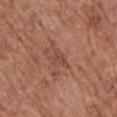Captured during whole-body skin photography for melanoma surveillance; the lesion was not biopsied. Imaged with white-light lighting. A lesion tile, about 15 mm wide, cut from a 3D total-body photograph. The lesion-visualizer software estimated a mean CIELAB color near L≈46 a*≈23 b*≈28, roughly 7 lightness units darker than nearby skin, and a lesion-to-skin contrast of about 5 (normalized; higher = more distinct). A female subject in their mid-70s. On the upper back.